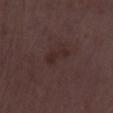notes: total-body-photography surveillance lesion; no biopsy
site: the leg
lesion size: about 3.5 mm
subject: female, aged 33–37
acquisition: total-body-photography crop, ~15 mm field of view
image-analysis metrics: a lesion–skin lightness drop of about 5 and a lesion-to-skin contrast of about 6 (normalized; higher = more distinct); a nevus-likeness score of about 0/100 and lesion-presence confidence of about 100/100